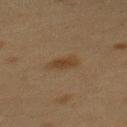Q: Was this lesion biopsied?
A: catalogued during a skin exam; not biopsied
Q: Automated lesion metrics?
A: a classifier nevus-likeness of about 90/100
Q: Patient demographics?
A: female, aged around 40
Q: What lighting was used for the tile?
A: cross-polarized illumination
Q: Where on the body is the lesion?
A: the upper back
Q: How was this image acquired?
A: ~15 mm crop, total-body skin-cancer survey